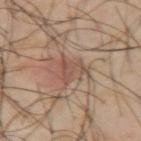Findings:
– notes: imaged on a skin check; not biopsied
– automated lesion analysis: a footprint of about 5 mm² and two-axis asymmetry of about 0.7; a lesion color around L≈51 a*≈19 b*≈27 in CIELAB, about 8 CIELAB-L* units darker than the surrounding skin, and a normalized lesion–skin contrast near 6; a nevus-likeness score of about 0/100 and lesion-presence confidence of about 65/100
– site: the right upper arm
– subject: male, aged 38–42
– lighting: cross-polarized illumination
– lesion size: ≈3 mm
– image source: ~15 mm tile from a whole-body skin photo